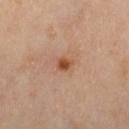A lesion tile, about 15 mm wide, cut from a 3D total-body photograph.
The lesion's longest dimension is about 2 mm.
The lesion is located on the left thigh.
Automated image analysis of the tile measured a lesion area of about 3 mm², a shape eccentricity near 0.65, and a shape-asymmetry score of about 0.3 (0 = symmetric). The analysis additionally found a border-irregularity rating of about 2.5/10, a within-lesion color-variation index near 2/10, and radial color variation of about 0.5. It also reported a classifier nevus-likeness of about 85/100.
The tile uses cross-polarized illumination.
A female subject, in their mid-50s.